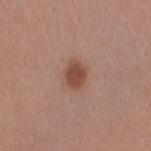* biopsy status · imaged on a skin check; not biopsied
* TBP lesion metrics · an area of roughly 5 mm² and a symmetry-axis asymmetry near 0.25; a border-irregularity index near 2/10 and peripheral color asymmetry of about 0.5
* size · ≈3 mm
* lighting · white-light illumination
* image source · 15 mm crop, total-body photography
* subject · male, in their 30s
* site · the right forearm